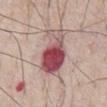The subject is a male roughly 70 years of age.
On the chest.
A roughly 15 mm field-of-view crop from a total-body skin photograph.